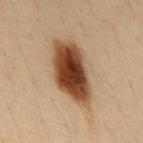follow-up: no biopsy performed (imaged during a skin exam)
image source: ~15 mm crop, total-body skin-cancer survey
lesion size: ~7 mm (longest diameter)
location: the mid back
image-analysis metrics: a lesion area of about 22 mm² and two-axis asymmetry of about 0.2; a color-variation rating of about 7/10 and peripheral color asymmetry of about 2
subject: female, approximately 50 years of age